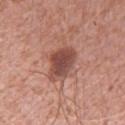Impression: Captured during whole-body skin photography for melanoma surveillance; the lesion was not biopsied. Clinical summary: Captured under white-light illumination. The lesion is located on the arm. Cropped from a whole-body photographic skin survey; the tile spans about 15 mm. A female patient, aged 38–42. The lesion's longest dimension is about 5 mm.